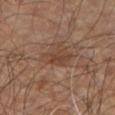biopsy status = total-body-photography surveillance lesion; no biopsy
subject = male, aged approximately 60
lesion diameter = ≈4 mm
acquisition = ~15 mm tile from a whole-body skin photo
illumination = cross-polarized
anatomic site = the right leg